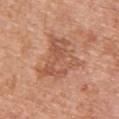Part of a total-body skin-imaging series; this lesion was reviewed on a skin check and was not flagged for biopsy.